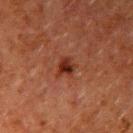{"biopsy_status": "not biopsied; imaged during a skin examination", "lighting": "cross-polarized", "lesion_size": {"long_diameter_mm_approx": 2.5}, "patient": {"sex": "male", "age_approx": 60}, "image": {"source": "total-body photography crop", "field_of_view_mm": 15}, "site": "arm"}